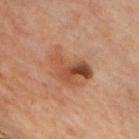Notes:
- workup: total-body-photography surveillance lesion; no biopsy
- subject: male, aged around 55
- imaging modality: ~15 mm crop, total-body skin-cancer survey
- diameter: ~5 mm (longest diameter)
- location: the chest